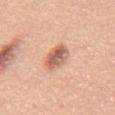Clinical impression: Recorded during total-body skin imaging; not selected for excision or biopsy. Clinical summary: The patient is a male aged 48 to 52. This is a white-light tile. A 15 mm close-up tile from a total-body photography series done for melanoma screening. The lesion is on the abdomen. About 3.5 mm across.